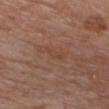Captured during whole-body skin photography for melanoma surveillance; the lesion was not biopsied.
The lesion's longest dimension is about 3 mm.
This image is a 15 mm lesion crop taken from a total-body photograph.
A female subject, aged around 75.
This is a white-light tile.
An algorithmic analysis of the crop reported a mean CIELAB color near L≈44 a*≈20 b*≈29, roughly 4 lightness units darker than nearby skin, and a normalized border contrast of about 4.5. The software also gave a border-irregularity rating of about 5/10 and peripheral color asymmetry of about 0. It also reported an automated nevus-likeness rating near 0 out of 100 and a detector confidence of about 100 out of 100 that the crop contains a lesion.
Located on the chest.